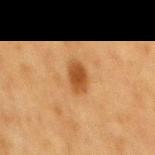| key | value |
|---|---|
| workup | total-body-photography surveillance lesion; no biopsy |
| lesion size | ≈3.5 mm |
| automated metrics | an automated nevus-likeness rating near 100 out of 100 |
| location | the mid back |
| patient | male, aged approximately 75 |
| acquisition | 15 mm crop, total-body photography |
| illumination | cross-polarized |